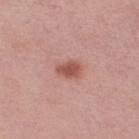Measured at roughly 3 mm in maximum diameter. Automated tile analysis of the lesion measured a footprint of about 4.5 mm² and an outline eccentricity of about 0.75 (0 = round, 1 = elongated). The analysis additionally found a border-irregularity index near 2.5/10 and a within-lesion color-variation index near 1.5/10. A female patient aged around 65. Captured under white-light illumination. A close-up tile cropped from a whole-body skin photograph, about 15 mm across. The lesion is on the right thigh.